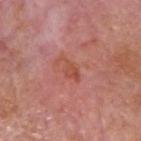Longest diameter approximately 3 mm.
A 15 mm crop from a total-body photograph taken for skin-cancer surveillance.
Located on the head or neck.
Automated image analysis of the tile measured a color-variation rating of about 1.5/10 and radial color variation of about 0.5. And it measured an automated nevus-likeness rating near 0 out of 100 and lesion-presence confidence of about 100/100.
A male patient, about 75 years old.
Imaged with white-light lighting.